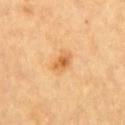Imaged during a routine full-body skin examination; the lesion was not biopsied and no histopathology is available. Automated tile analysis of the lesion measured an average lesion color of about L≈63 a*≈24 b*≈45 (CIELAB), roughly 10 lightness units darker than nearby skin, and a lesion-to-skin contrast of about 7 (normalized; higher = more distinct). The analysis additionally found internal color variation of about 5 on a 0–10 scale and a peripheral color-asymmetry measure near 1.5. A roughly 15 mm field-of-view crop from a total-body skin photograph. The patient is a male aged 83 or over. Measured at roughly 3 mm in maximum diameter. From the chest.